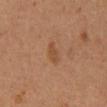workup=total-body-photography surveillance lesion; no biopsy | image-analysis metrics=a footprint of about 3 mm² and a symmetry-axis asymmetry near 0.4; a classifier nevus-likeness of about 20/100 and lesion-presence confidence of about 100/100 | image source=~15 mm tile from a whole-body skin photo | size=≈2.5 mm | subject=male, aged around 60 | anatomic site=the mid back.